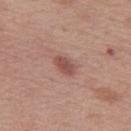<record>
  <biopsy_status>not biopsied; imaged during a skin examination</biopsy_status>
  <patient>
    <sex>female</sex>
    <age_approx>50</age_approx>
  </patient>
  <lesion_size>
    <long_diameter_mm_approx>3.0</long_diameter_mm_approx>
  </lesion_size>
  <lighting>white-light</lighting>
  <automated_metrics>
    <vs_skin_darker_L>10.0</vs_skin_darker_L>
    <vs_skin_contrast_norm>7.5</vs_skin_contrast_norm>
    <border_irregularity_0_10>2.0</border_irregularity_0_10>
    <color_variation_0_10>2.5</color_variation_0_10>
    <peripheral_color_asymmetry>0.5</peripheral_color_asymmetry>
    <lesion_detection_confidence_0_100>100</lesion_detection_confidence_0_100>
  </automated_metrics>
  <image>
    <source>total-body photography crop</source>
    <field_of_view_mm>15</field_of_view_mm>
  </image>
  <site>leg</site>
</record>